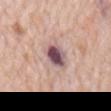The lesion was tiled from a total-body skin photograph and was not biopsied.
On the mid back.
Captured under white-light illumination.
This image is a 15 mm lesion crop taken from a total-body photograph.
A male subject, aged approximately 80.
The recorded lesion diameter is about 4 mm.